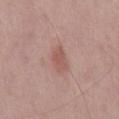follow-up — no biopsy performed (imaged during a skin exam) | tile lighting — white-light | subject — male, approximately 70 years of age | lesion diameter — ~3 mm (longest diameter) | imaging modality — ~15 mm tile from a whole-body skin photo | body site — the back.